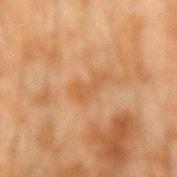This lesion was catalogued during total-body skin photography and was not selected for biopsy. About 3.5 mm across. The tile uses cross-polarized illumination. Located on the right forearm. A male patient, aged approximately 45. The lesion-visualizer software estimated an average lesion color of about L≈47 a*≈19 b*≈35 (CIELAB) and a normalized border contrast of about 5. The software also gave an automated nevus-likeness rating near 0 out of 100 and a detector confidence of about 100 out of 100 that the crop contains a lesion. A 15 mm close-up extracted from a 3D total-body photography capture.